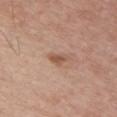  biopsy_status: not biopsied; imaged during a skin examination
  image:
    source: total-body photography crop
    field_of_view_mm: 15
  site: chest
  automated_metrics:
    cielab_L: 54
    cielab_a: 20
    cielab_b: 30
    vs_skin_darker_L: 10.0
    vs_skin_contrast_norm: 7.0
    border_irregularity_0_10: 3.0
    color_variation_0_10: 1.5
    peripheral_color_asymmetry: 0.5
    nevus_likeness_0_100: 40
    lesion_detection_confidence_0_100: 100
  lesion_size:
    long_diameter_mm_approx: 2.5
  lighting: white-light
  patient:
    sex: female
    age_approx: 30